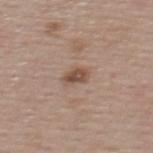Q: Is there a histopathology result?
A: no biopsy performed (imaged during a skin exam)
Q: What is the imaging modality?
A: total-body-photography crop, ~15 mm field of view
Q: How large is the lesion?
A: ~3 mm (longest diameter)
Q: Where on the body is the lesion?
A: the upper back
Q: Automated lesion metrics?
A: a mean CIELAB color near L≈50 a*≈18 b*≈27, about 10 CIELAB-L* units darker than the surrounding skin, and a normalized border contrast of about 8; a nevus-likeness score of about 80/100 and lesion-presence confidence of about 100/100
Q: Patient demographics?
A: female, aged around 55